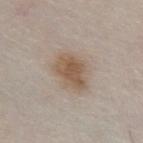Longest diameter approximately 5 mm. A region of skin cropped from a whole-body photographic capture, roughly 15 mm wide. Imaged with white-light lighting. The patient is a male roughly 80 years of age. Automated tile analysis of the lesion measured a shape eccentricity near 0.75. The analysis additionally found a lesion–skin lightness drop of about 10 and a lesion-to-skin contrast of about 8 (normalized; higher = more distinct). The software also gave a color-variation rating of about 3/10 and a peripheral color-asymmetry measure near 1. The lesion is on the abdomen.